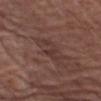Part of a total-body skin-imaging series; this lesion was reviewed on a skin check and was not flagged for biopsy. The tile uses white-light illumination. A male subject approximately 75 years of age. Located on the arm. The total-body-photography lesion software estimated a footprint of about 5 mm², an outline eccentricity of about 0.9 (0 = round, 1 = elongated), and two-axis asymmetry of about 0.4. A close-up tile cropped from a whole-body skin photograph, about 15 mm across.